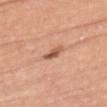Q: Is there a histopathology result?
A: catalogued during a skin exam; not biopsied
Q: What kind of image is this?
A: 15 mm crop, total-body photography
Q: How large is the lesion?
A: ~2.5 mm (longest diameter)
Q: What is the anatomic site?
A: the chest
Q: What are the patient's age and sex?
A: female, aged 73 to 77